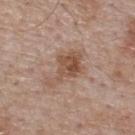<case>
  <biopsy_status>not biopsied; imaged during a skin examination</biopsy_status>
  <site>upper back</site>
  <lesion_size>
    <long_diameter_mm_approx>5.0</long_diameter_mm_approx>
  </lesion_size>
  <patient>
    <sex>male</sex>
    <age_approx>55</age_approx>
  </patient>
  <automated_metrics>
    <border_irregularity_0_10>6.0</border_irregularity_0_10>
  </automated_metrics>
  <image>
    <source>total-body photography crop</source>
    <field_of_view_mm>15</field_of_view_mm>
  </image>
</case>